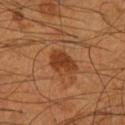follow-up: no biopsy performed (imaged during a skin exam) | illumination: cross-polarized | patient: male, aged 53–57 | automated lesion analysis: a lesion area of about 7 mm², an outline eccentricity of about 0.75 (0 = round, 1 = elongated), and a symmetry-axis asymmetry near 0.25; a nevus-likeness score of about 90/100 and a detector confidence of about 100 out of 100 that the crop contains a lesion | image source: total-body-photography crop, ~15 mm field of view | anatomic site: the right lower leg | size: ≈4 mm.